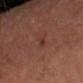notes: imaged on a skin check; not biopsied | lighting: cross-polarized | body site: the right forearm | lesion size: ~3 mm (longest diameter) | patient: male, aged approximately 45 | acquisition: 15 mm crop, total-body photography.